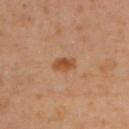A 15 mm close-up tile from a total-body photography series done for melanoma screening. Located on the upper back. An algorithmic analysis of the crop reported a footprint of about 4 mm² and a shape-asymmetry score of about 0.25 (0 = symmetric). The software also gave a border-irregularity index near 2.5/10, internal color variation of about 2.5 on a 0–10 scale, and radial color variation of about 1. The analysis additionally found a classifier nevus-likeness of about 90/100 and a detector confidence of about 100 out of 100 that the crop contains a lesion. The subject is a female aged 38–42. Measured at roughly 3 mm in maximum diameter. The tile uses cross-polarized illumination.